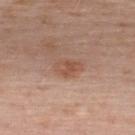{"automated_metrics": {"area_mm2_approx": 5.5, "eccentricity": 0.75, "shape_asymmetry": 0.25, "cielab_L": 52, "cielab_a": 21, "cielab_b": 30, "vs_skin_darker_L": 8.0, "vs_skin_contrast_norm": 6.5, "border_irregularity_0_10": 2.0, "color_variation_0_10": 3.5, "peripheral_color_asymmetry": 1.0, "nevus_likeness_0_100": 15, "lesion_detection_confidence_0_100": 100}, "lighting": "white-light", "image": {"source": "total-body photography crop", "field_of_view_mm": 15}, "site": "upper back", "patient": {"sex": "male", "age_approx": 40}, "lesion_size": {"long_diameter_mm_approx": 3.0}}